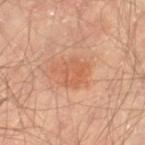This lesion was catalogued during total-body skin photography and was not selected for biopsy.
The lesion-visualizer software estimated a footprint of about 9.5 mm² and a symmetry-axis asymmetry near 0.3. The software also gave an average lesion color of about L≈58 a*≈25 b*≈34 (CIELAB), about 7 CIELAB-L* units darker than the surrounding skin, and a normalized lesion–skin contrast near 5.5.
On the left thigh.
This is a cross-polarized tile.
A male patient, aged around 65.
The lesion's longest dimension is about 4 mm.
A lesion tile, about 15 mm wide, cut from a 3D total-body photograph.